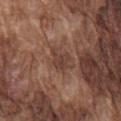Q: Is there a histopathology result?
A: imaged on a skin check; not biopsied
Q: How was this image acquired?
A: total-body-photography crop, ~15 mm field of view
Q: Where on the body is the lesion?
A: the front of the torso
Q: Lesion size?
A: about 3.5 mm
Q: Patient demographics?
A: male, roughly 75 years of age
Q: What lighting was used for the tile?
A: white-light
Q: What did automated image analysis measure?
A: a lesion color around L≈40 a*≈19 b*≈25 in CIELAB, roughly 7 lightness units darker than nearby skin, and a normalized border contrast of about 6.5; a border-irregularity index near 4.5/10 and a peripheral color-asymmetry measure near 0.5; a nevus-likeness score of about 0/100 and lesion-presence confidence of about 90/100